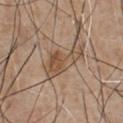The lesion was photographed on a routine skin check and not biopsied; there is no pathology result. The lesion is located on the chest. Cropped from a whole-body photographic skin survey; the tile spans about 15 mm. Automated tile analysis of the lesion measured a mean CIELAB color near L≈51 a*≈16 b*≈30, roughly 9 lightness units darker than nearby skin, and a normalized border contrast of about 7. The analysis additionally found a border-irregularity rating of about 5.5/10, a within-lesion color-variation index near 5.5/10, and radial color variation of about 2. It also reported a nevus-likeness score of about 75/100 and a lesion-detection confidence of about 100/100. Longest diameter approximately 6 mm. A male subject aged 63–67. Imaged with white-light lighting.